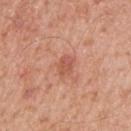notes = imaged on a skin check; not biopsied | diameter = ≈2.5 mm | anatomic site = the left upper arm | image = ~15 mm tile from a whole-body skin photo | patient = male, approximately 55 years of age | image-analysis metrics = a footprint of about 4 mm² and a symmetry-axis asymmetry near 0.25; a mean CIELAB color near L≈56 a*≈27 b*≈32 and about 9 CIELAB-L* units darker than the surrounding skin; a border-irregularity index near 2.5/10 and internal color variation of about 4 on a 0–10 scale | lighting = white-light illumination.